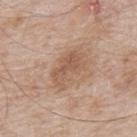No biopsy was performed on this lesion — it was imaged during a full skin examination and was not determined to be concerning.
Approximately 5 mm at its widest.
On the mid back.
A male subject aged 63 to 67.
A close-up tile cropped from a whole-body skin photograph, about 15 mm across.
The lesion-visualizer software estimated border irregularity of about 4.5 on a 0–10 scale, internal color variation of about 3 on a 0–10 scale, and peripheral color asymmetry of about 1. And it measured a nevus-likeness score of about 0/100 and a lesion-detection confidence of about 100/100.